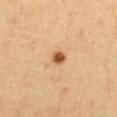{
  "biopsy_status": "not biopsied; imaged during a skin examination",
  "lighting": "cross-polarized",
  "patient": {
    "sex": "female",
    "age_approx": 45
  },
  "lesion_size": {
    "long_diameter_mm_approx": 2.0
  },
  "site": "chest",
  "image": {
    "source": "total-body photography crop",
    "field_of_view_mm": 15
  }
}